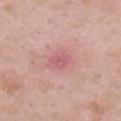Context:
The tile uses white-light illumination. The lesion-visualizer software estimated a lesion area of about 4.5 mm², a shape eccentricity near 0.7, and a symmetry-axis asymmetry near 0.25. The software also gave a color-variation rating of about 2.5/10 and a peripheral color-asymmetry measure near 0.5. Located on the arm. The subject is a male aged 53 to 57. A 15 mm close-up extracted from a 3D total-body photography capture. The recorded lesion diameter is about 3 mm.